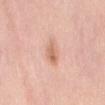Case summary:
- biopsy status — no biopsy performed (imaged during a skin exam)
- patient — female, in their 50s
- image — total-body-photography crop, ~15 mm field of view
- location — the abdomen